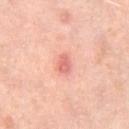Part of a total-body skin-imaging series; this lesion was reviewed on a skin check and was not flagged for biopsy.
This image is a 15 mm lesion crop taken from a total-body photograph.
The recorded lesion diameter is about 2.5 mm.
A female patient, aged approximately 50.
This is a cross-polarized tile.
The lesion is located on the left thigh.